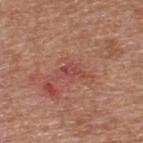{
  "biopsy_status": "not biopsied; imaged during a skin examination",
  "image": {
    "source": "total-body photography crop",
    "field_of_view_mm": 15
  },
  "patient": {
    "sex": "male",
    "age_approx": 65
  },
  "lesion_size": {
    "long_diameter_mm_approx": 3.0
  },
  "lighting": "white-light",
  "site": "upper back",
  "automated_metrics": {
    "border_irregularity_0_10": 4.5,
    "color_variation_0_10": 0.0,
    "nevus_likeness_0_100": 0
  }
}